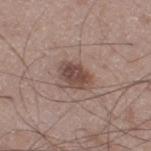Notes:
* workup: catalogued during a skin exam; not biopsied
* size: about 4 mm
* image: ~15 mm tile from a whole-body skin photo
* tile lighting: white-light
* subject: male, aged 48 to 52
* body site: the right thigh